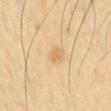Imaged during a routine full-body skin examination; the lesion was not biopsied and no histopathology is available. On the left upper arm. A male subject in their mid- to late 40s. This image is a 15 mm lesion crop taken from a total-body photograph.